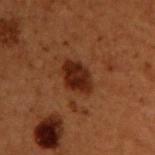{
  "biopsy_status": "not biopsied; imaged during a skin examination",
  "lighting": "cross-polarized",
  "site": "upper back",
  "image": {
    "source": "total-body photography crop",
    "field_of_view_mm": 15
  },
  "lesion_size": {
    "long_diameter_mm_approx": 4.5
  },
  "patient": {
    "sex": "male",
    "age_approx": 50
  },
  "automated_metrics": {
    "area_mm2_approx": 8.5,
    "eccentricity": 0.75,
    "shape_asymmetry": 0.15,
    "cielab_L": 20,
    "cielab_a": 20,
    "cielab_b": 24,
    "vs_skin_contrast_norm": 11.0,
    "nevus_likeness_0_100": 90
  }
}